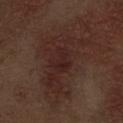{
  "biopsy_status": "not biopsied; imaged during a skin examination"
}